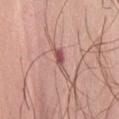Q: Automated lesion metrics?
A: a lesion area of about 3.5 mm², an outline eccentricity of about 0.95 (0 = round, 1 = elongated), and two-axis asymmetry of about 0.5; a border-irregularity index near 6/10, a color-variation rating of about 1/10, and peripheral color asymmetry of about 0
Q: Where on the body is the lesion?
A: the front of the torso
Q: What is the imaging modality?
A: ~15 mm crop, total-body skin-cancer survey
Q: What are the patient's age and sex?
A: female, about 55 years old
Q: How large is the lesion?
A: ~4 mm (longest diameter)
Q: What lighting was used for the tile?
A: white-light